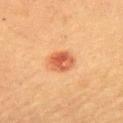follow-up: no biopsy performed (imaged during a skin exam) | illumination: cross-polarized illumination | image: total-body-photography crop, ~15 mm field of view | lesion diameter: about 3.5 mm | TBP lesion metrics: an outline eccentricity of about 0.55 (0 = round, 1 = elongated) and a symmetry-axis asymmetry near 0.2; a border-irregularity rating of about 1.5/10, a within-lesion color-variation index near 5.5/10, and radial color variation of about 2; a nevus-likeness score of about 100/100 and a lesion-detection confidence of about 100/100 | site: the upper back | subject: female, in their 60s.